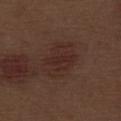Clinical impression:
This lesion was catalogued during total-body skin photography and was not selected for biopsy.
Image and clinical context:
The recorded lesion diameter is about 5 mm. The tile uses white-light illumination. A close-up tile cropped from a whole-body skin photograph, about 15 mm across. A male patient approximately 70 years of age. The lesion is located on the leg.